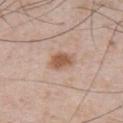Assessment: The lesion was tiled from a total-body skin photograph and was not biopsied. Background: A 15 mm crop from a total-body photograph taken for skin-cancer surveillance. The patient is a male about 55 years old. The total-body-photography lesion software estimated a lesion area of about 6 mm² and an outline eccentricity of about 0.7 (0 = round, 1 = elongated). The analysis additionally found a mean CIELAB color near L≈57 a*≈19 b*≈30, a lesion–skin lightness drop of about 11, and a normalized lesion–skin contrast near 8. The software also gave a border-irregularity rating of about 2/10 and radial color variation of about 1. The tile uses white-light illumination. The lesion is on the chest.